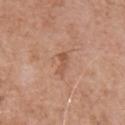biopsy status: no biopsy performed (imaged during a skin exam) | site: the front of the torso | subject: male, aged 63–67 | imaging modality: total-body-photography crop, ~15 mm field of view.